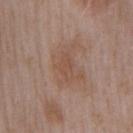Case summary:
• site — the right upper arm
• automated metrics — a lesion area of about 4 mm² and two-axis asymmetry of about 0.3; a lesion–skin lightness drop of about 5 and a lesion-to-skin contrast of about 4.5 (normalized; higher = more distinct); a classifier nevus-likeness of about 0/100 and a detector confidence of about 100 out of 100 that the crop contains a lesion
• tile lighting — white-light illumination
• image — total-body-photography crop, ~15 mm field of view
• lesion size — ~3 mm (longest diameter)
• patient — male, aged 53 to 57